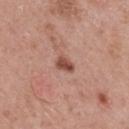Case summary:
– notes — imaged on a skin check; not biopsied
– acquisition — ~15 mm tile from a whole-body skin photo
– automated metrics — a shape-asymmetry score of about 0.25 (0 = symmetric); a lesion color around L≈49 a*≈24 b*≈27 in CIELAB, a lesion–skin lightness drop of about 13, and a normalized border contrast of about 9
– subject — male, in their mid-60s
– anatomic site — the upper back
– diameter — ~2.5 mm (longest diameter)